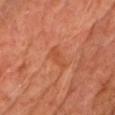Assessment:
Captured during whole-body skin photography for melanoma surveillance; the lesion was not biopsied.
Image and clinical context:
On the upper back. Approximately 3 mm at its widest. A 15 mm close-up tile from a total-body photography series done for melanoma screening. Imaged with cross-polarized lighting. A male patient, aged around 60.